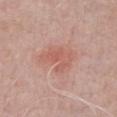Impression: Recorded during total-body skin imaging; not selected for excision or biopsy. Context: The subject is a male in their mid-60s. The lesion is on the chest. Cropped from a total-body skin-imaging series; the visible field is about 15 mm.